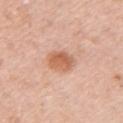Assessment:
No biopsy was performed on this lesion — it was imaged during a full skin examination and was not determined to be concerning.
Acquisition and patient details:
Captured under white-light illumination. The patient is a female aged around 50. A region of skin cropped from a whole-body photographic capture, roughly 15 mm wide. On the arm. Measured at roughly 4 mm in maximum diameter. The total-body-photography lesion software estimated a footprint of about 7.5 mm², an outline eccentricity of about 0.75 (0 = round, 1 = elongated), and a symmetry-axis asymmetry near 0.1. And it measured a mean CIELAB color near L≈62 a*≈25 b*≈34, about 11 CIELAB-L* units darker than the surrounding skin, and a lesion-to-skin contrast of about 8 (normalized; higher = more distinct). The analysis additionally found a border-irregularity rating of about 1.5/10, a color-variation rating of about 3.5/10, and a peripheral color-asymmetry measure near 1. It also reported an automated nevus-likeness rating near 95 out of 100 and a detector confidence of about 100 out of 100 that the crop contains a lesion.